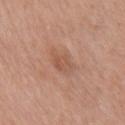This lesion was catalogued during total-body skin photography and was not selected for biopsy. Imaged with white-light lighting. A female subject, roughly 60 years of age. Located on the chest. Automated tile analysis of the lesion measured an average lesion color of about L≈54 a*≈22 b*≈30 (CIELAB), about 7 CIELAB-L* units darker than the surrounding skin, and a normalized lesion–skin contrast near 5. Cropped from a whole-body photographic skin survey; the tile spans about 15 mm.